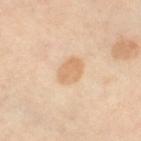Clinical impression: Part of a total-body skin-imaging series; this lesion was reviewed on a skin check and was not flagged for biopsy. Image and clinical context: A 15 mm close-up tile from a total-body photography series done for melanoma screening. Located on the leg. A female patient, about 50 years old. Measured at roughly 3 mm in maximum diameter.